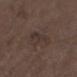Q: Is there a histopathology result?
A: total-body-photography surveillance lesion; no biopsy
Q: What is the imaging modality?
A: ~15 mm tile from a whole-body skin photo
Q: Lesion location?
A: the left lower leg
Q: Who is the patient?
A: male, in their mid-70s
Q: Illumination type?
A: white-light illumination
Q: How large is the lesion?
A: about 5 mm